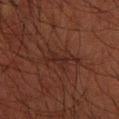Captured during whole-body skin photography for melanoma surveillance; the lesion was not biopsied.
Captured under cross-polarized illumination.
The lesion-visualizer software estimated a symmetry-axis asymmetry near 0.5. It also reported a lesion color around L≈19 a*≈17 b*≈19 in CIELAB, a lesion–skin lightness drop of about 5, and a lesion-to-skin contrast of about 6.5 (normalized; higher = more distinct). The analysis additionally found border irregularity of about 7.5 on a 0–10 scale and a within-lesion color-variation index near 1.5/10. The software also gave a nevus-likeness score of about 0/100.
The patient is a male aged approximately 60.
The lesion is located on the right forearm.
Cropped from a total-body skin-imaging series; the visible field is about 15 mm.
Approximately 4.5 mm at its widest.